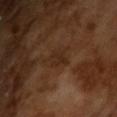| feature | finding |
|---|---|
| biopsy status | total-body-photography surveillance lesion; no biopsy |
| imaging modality | ~15 mm crop, total-body skin-cancer survey |
| illumination | cross-polarized illumination |
| automated lesion analysis | a border-irregularity index near 3.5/10, a color-variation rating of about 1.5/10, and radial color variation of about 0.5; lesion-presence confidence of about 100/100 |
| diameter | ~3 mm (longest diameter) |
| patient | male, aged approximately 65 |